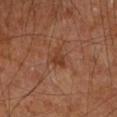Clinical impression: Captured during whole-body skin photography for melanoma surveillance; the lesion was not biopsied. Image and clinical context: The lesion is on the left forearm. The total-body-photography lesion software estimated a footprint of about 3.5 mm², an eccentricity of roughly 0.65, and a symmetry-axis asymmetry near 0.5. And it measured a lesion color around L≈37 a*≈23 b*≈31 in CIELAB, about 7 CIELAB-L* units darker than the surrounding skin, and a normalized border contrast of about 6.5. The software also gave a border-irregularity index near 4.5/10 and peripheral color asymmetry of about 0.5. And it measured a classifier nevus-likeness of about 0/100 and a detector confidence of about 100 out of 100 that the crop contains a lesion. The lesion's longest dimension is about 2.5 mm. Captured under cross-polarized illumination. The subject is a male roughly 85 years of age or older. A 15 mm close-up extracted from a 3D total-body photography capture.